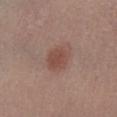{"biopsy_status": "not biopsied; imaged during a skin examination", "patient": {"sex": "female", "age_approx": 50}, "lighting": "white-light", "lesion_size": {"long_diameter_mm_approx": 3.5}, "automated_metrics": {"area_mm2_approx": 8.0, "eccentricity": 0.45, "shape_asymmetry": 0.2, "cielab_L": 48, "cielab_a": 21, "cielab_b": 24, "vs_skin_darker_L": 8.0, "vs_skin_contrast_norm": 6.5, "border_irregularity_0_10": 2.0, "peripheral_color_asymmetry": 1.0, "lesion_detection_confidence_0_100": 100}, "image": {"source": "total-body photography crop", "field_of_view_mm": 15}, "site": "left lower leg"}